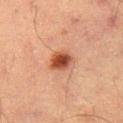About 3 mm across.
Captured under cross-polarized illumination.
A male subject, roughly 65 years of age.
A lesion tile, about 15 mm wide, cut from a 3D total-body photograph.
Located on the right thigh.